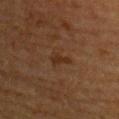| key | value |
|---|---|
| notes | total-body-photography surveillance lesion; no biopsy |
| image source | 15 mm crop, total-body photography |
| lesion size | ~3 mm (longest diameter) |
| illumination | cross-polarized |
| body site | the upper back |
| automated lesion analysis | a lesion area of about 3 mm², a shape eccentricity near 0.9, and a shape-asymmetry score of about 0.45 (0 = symmetric); a lesion–skin lightness drop of about 5 and a lesion-to-skin contrast of about 6.5 (normalized; higher = more distinct); internal color variation of about 0.5 on a 0–10 scale and peripheral color asymmetry of about 0; a detector confidence of about 100 out of 100 that the crop contains a lesion |
| patient | female, aged approximately 55 |